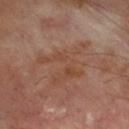notes = no biopsy performed (imaged during a skin exam).